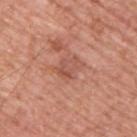Q: Was a biopsy performed?
A: no biopsy performed (imaged during a skin exam)
Q: What kind of image is this?
A: total-body-photography crop, ~15 mm field of view
Q: How was the tile lit?
A: white-light
Q: Lesion size?
A: ≈3.5 mm
Q: Who is the patient?
A: male, about 55 years old
Q: What is the anatomic site?
A: the upper back